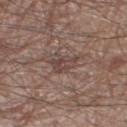follow-up: total-body-photography surveillance lesion; no biopsy | size: ≈3.5 mm | lighting: white-light | image source: total-body-photography crop, ~15 mm field of view | body site: the right thigh | subject: male, aged around 80.